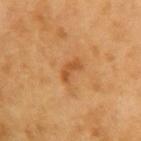notes = catalogued during a skin exam; not biopsied | image source = 15 mm crop, total-body photography | lesion size = ~3 mm (longest diameter) | patient = male, about 60 years old | anatomic site = the left upper arm | lighting = cross-polarized.